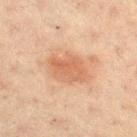Recorded during total-body skin imaging; not selected for excision or biopsy. The total-body-photography lesion software estimated an average lesion color of about L≈52 a*≈20 b*≈30 (CIELAB), about 8 CIELAB-L* units darker than the surrounding skin, and a lesion-to-skin contrast of about 6 (normalized; higher = more distinct). Imaged with cross-polarized lighting. Approximately 5 mm at its widest. This image is a 15 mm lesion crop taken from a total-body photograph. The subject is a female approximately 40 years of age. The lesion is on the right thigh.